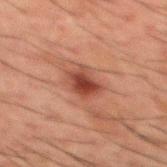No biopsy was performed on this lesion — it was imaged during a full skin examination and was not determined to be concerning. Imaged with cross-polarized lighting. Approximately 3 mm at its widest. The subject is a male approximately 50 years of age. The lesion-visualizer software estimated a footprint of about 6.5 mm², a shape eccentricity near 0.5, and a shape-asymmetry score of about 0.25 (0 = symmetric). It also reported a border-irregularity rating of about 3/10, internal color variation of about 3.5 on a 0–10 scale, and a peripheral color-asymmetry measure near 1. A 15 mm close-up extracted from a 3D total-body photography capture. From the mid back.